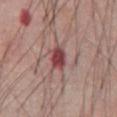biopsy status: total-body-photography surveillance lesion; no biopsy
location: the abdomen
tile lighting: white-light illumination
imaging modality: total-body-photography crop, ~15 mm field of view
patient: male, aged 53–57
automated metrics: an area of roughly 6 mm², a shape eccentricity near 0.75, and two-axis asymmetry of about 0.2; a lesion color around L≈45 a*≈27 b*≈20 in CIELAB